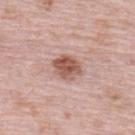Clinical impression:
This lesion was catalogued during total-body skin photography and was not selected for biopsy.
Image and clinical context:
A 15 mm crop from a total-body photograph taken for skin-cancer surveillance. On the upper back. Captured under white-light illumination. A female subject aged approximately 65.